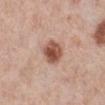• follow-up · catalogued during a skin exam; not biopsied
• illumination · white-light illumination
• diameter · about 3 mm
• patient · female, about 65 years old
• image · ~15 mm tile from a whole-body skin photo
• anatomic site · the right lower leg